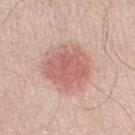This lesion was catalogued during total-body skin photography and was not selected for biopsy. The recorded lesion diameter is about 5.5 mm. Cropped from a whole-body photographic skin survey; the tile spans about 15 mm. From the leg. A male subject approximately 70 years of age.